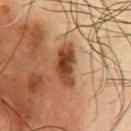Impression: Recorded during total-body skin imaging; not selected for excision or biopsy. Clinical summary: On the chest. This image is a 15 mm lesion crop taken from a total-body photograph. The total-body-photography lesion software estimated a footprint of about 8.5 mm², an outline eccentricity of about 0.9 (0 = round, 1 = elongated), and two-axis asymmetry of about 0.35. The software also gave an average lesion color of about L≈35 a*≈20 b*≈30 (CIELAB), a lesion–skin lightness drop of about 13, and a normalized lesion–skin contrast near 11. The analysis additionally found a border-irregularity index near 3.5/10. And it measured a nevus-likeness score of about 25/100 and a lesion-detection confidence of about 100/100. Measured at roughly 4.5 mm in maximum diameter. A male patient, aged 58 to 62.